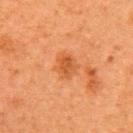Part of a total-body skin-imaging series; this lesion was reviewed on a skin check and was not flagged for biopsy. The tile uses cross-polarized illumination. A close-up tile cropped from a whole-body skin photograph, about 15 mm across. A female subject, aged around 60. About 3.5 mm across. The lesion is on the right upper arm.